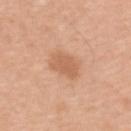  biopsy_status: not biopsied; imaged during a skin examination
  lighting: white-light
  lesion_size:
    long_diameter_mm_approx: 3.0
  patient:
    sex: male
    age_approx: 45
  site: upper back
  automated_metrics:
    border_irregularity_0_10: 2.0
    color_variation_0_10: 1.5
    peripheral_color_asymmetry: 0.5
  image:
    source: total-body photography crop
    field_of_view_mm: 15